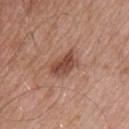| feature | finding |
|---|---|
| notes | catalogued during a skin exam; not biopsied |
| patient | male, aged 53 to 57 |
| image | ~15 mm tile from a whole-body skin photo |
| automated metrics | a footprint of about 6.5 mm² and a shape-asymmetry score of about 0.2 (0 = symmetric); a lesion color around L≈47 a*≈23 b*≈29 in CIELAB, roughly 12 lightness units darker than nearby skin, and a normalized border contrast of about 8.5 |
| lesion size | ~3.5 mm (longest diameter) |
| site | the back |